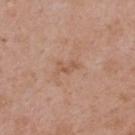Assessment: The lesion was photographed on a routine skin check and not biopsied; there is no pathology result. Clinical summary: The total-body-photography lesion software estimated a nevus-likeness score of about 0/100 and a lesion-detection confidence of about 100/100. The lesion is located on the upper back. Longest diameter approximately 2.5 mm. The subject is a female aged around 40. A roughly 15 mm field-of-view crop from a total-body skin photograph.